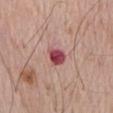notes: catalogued during a skin exam; not biopsied
tile lighting: white-light
site: the chest
patient: male, approximately 70 years of age
acquisition: total-body-photography crop, ~15 mm field of view
diameter: ≈2.5 mm